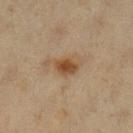The lesion was tiled from a total-body skin photograph and was not biopsied. Captured under cross-polarized illumination. A female patient, in their 40s. An algorithmic analysis of the crop reported a border-irregularity index near 2/10, internal color variation of about 2.5 on a 0–10 scale, and radial color variation of about 1. The software also gave a nevus-likeness score of about 90/100 and a detector confidence of about 100 out of 100 that the crop contains a lesion. Located on the left lower leg. Longest diameter approximately 2.5 mm. A close-up tile cropped from a whole-body skin photograph, about 15 mm across.